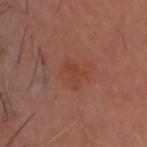<tbp_lesion>
  <biopsy_status>not biopsied; imaged during a skin examination</biopsy_status>
  <image>
    <source>total-body photography crop</source>
    <field_of_view_mm>15</field_of_view_mm>
  </image>
  <site>head or neck</site>
  <patient>
    <sex>male</sex>
    <age_approx>65</age_approx>
  </patient>
  <lesion_size>
    <long_diameter_mm_approx>3.0</long_diameter_mm_approx>
  </lesion_size>
</tbp_lesion>